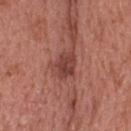workup=catalogued during a skin exam; not biopsied
body site=the upper back
lesion diameter=about 3 mm
patient=female, in their 60s
image=15 mm crop, total-body photography
TBP lesion metrics=a lesion area of about 6 mm²; a nevus-likeness score of about 30/100 and a detector confidence of about 100 out of 100 that the crop contains a lesion